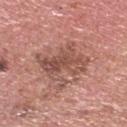Captured during whole-body skin photography for melanoma surveillance; the lesion was not biopsied. The total-body-photography lesion software estimated an eccentricity of roughly 0.65 and two-axis asymmetry of about 0.35. The software also gave a mean CIELAB color near L≈51 a*≈23 b*≈26, roughly 10 lightness units darker than nearby skin, and a normalized border contrast of about 7. And it measured border irregularity of about 7 on a 0–10 scale, a within-lesion color-variation index near 5.5/10, and peripheral color asymmetry of about 2. From the head or neck. This is a white-light tile. Cropped from a whole-body photographic skin survey; the tile spans about 15 mm. The recorded lesion diameter is about 5.5 mm. The patient is a male aged 63–67.